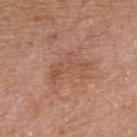Clinical impression:
Captured during whole-body skin photography for melanoma surveillance; the lesion was not biopsied.
Acquisition and patient details:
The lesion is on the right forearm. A female subject, aged 58 to 62. A close-up tile cropped from a whole-body skin photograph, about 15 mm across. This is a white-light tile.